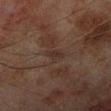- notes: no biopsy performed (imaged during a skin exam)